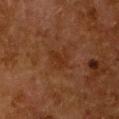<record>
<biopsy_status>not biopsied; imaged during a skin examination</biopsy_status>
<patient>
  <sex>female</sex>
  <age_approx>50</age_approx>
</patient>
<automated_metrics>
  <cielab_L>25</cielab_L>
  <cielab_a>19</cielab_a>
  <cielab_b>27</cielab_b>
  <vs_skin_contrast_norm>5.5</vs_skin_contrast_norm>
</automated_metrics>
<image>
  <source>total-body photography crop</source>
  <field_of_view_mm>15</field_of_view_mm>
</image>
<site>chest</site>
</record>